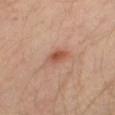workup=imaged on a skin check; not biopsied | subject=male, about 30 years old | image source=15 mm crop, total-body photography | body site=the left leg.